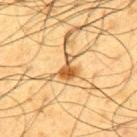biopsy status = total-body-photography surveillance lesion; no biopsy